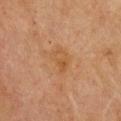workup = no biopsy performed (imaged during a skin exam)
body site = the chest
subject = female, roughly 55 years of age
image = total-body-photography crop, ~15 mm field of view
diameter = about 3 mm